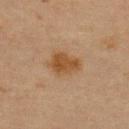This lesion was catalogued during total-body skin photography and was not selected for biopsy. The recorded lesion diameter is about 4 mm. A region of skin cropped from a whole-body photographic capture, roughly 15 mm wide. Automated image analysis of the tile measured a footprint of about 8.5 mm² and two-axis asymmetry of about 0.3. The analysis additionally found about 8 CIELAB-L* units darker than the surrounding skin and a normalized lesion–skin contrast near 8.5. And it measured an automated nevus-likeness rating near 90 out of 100 and lesion-presence confidence of about 100/100. The subject is a female roughly 45 years of age. The lesion is on the upper back.